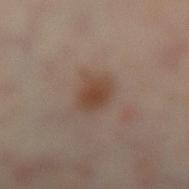biopsy_status: not biopsied; imaged during a skin examination
image:
  source: total-body photography crop
  field_of_view_mm: 15
lesion_size:
  long_diameter_mm_approx: 3.5
patient:
  sex: female
  age_approx: 35
lighting: cross-polarized
automated_metrics:
  area_mm2_approx: 7.5
  eccentricity: 0.7
  cielab_L: 41
  cielab_a: 16
  cielab_b: 26
  vs_skin_darker_L: 8.0
  vs_skin_contrast_norm: 8.0
  border_irregularity_0_10: 2.0
  peripheral_color_asymmetry: 0.5
site: left lower leg